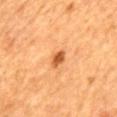Clinical impression: The lesion was photographed on a routine skin check and not biopsied; there is no pathology result. Background: A 15 mm close-up tile from a total-body photography series done for melanoma screening. From the back. A female patient, aged 53–57.